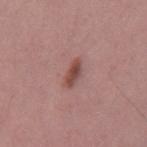No biopsy was performed on this lesion — it was imaged during a full skin examination and was not determined to be concerning.
The lesion is located on the mid back.
Approximately 3 mm at its widest.
A male subject about 35 years old.
Captured under white-light illumination.
The lesion-visualizer software estimated an average lesion color of about L≈47 a*≈23 b*≈23 (CIELAB) and a normalized lesion–skin contrast near 8.5.
Cropped from a whole-body photographic skin survey; the tile spans about 15 mm.